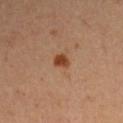Q: Is there a histopathology result?
A: catalogued during a skin exam; not biopsied
Q: How was this image acquired?
A: total-body-photography crop, ~15 mm field of view
Q: What lighting was used for the tile?
A: cross-polarized illumination
Q: Patient demographics?
A: male, in their mid- to late 60s
Q: Where on the body is the lesion?
A: the right upper arm
Q: Automated lesion metrics?
A: a mean CIELAB color near L≈42 a*≈23 b*≈33; a within-lesion color-variation index near 2/10 and a peripheral color-asymmetry measure near 0.5
Q: How large is the lesion?
A: ~2 mm (longest diameter)